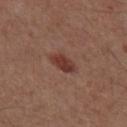Imaged during a routine full-body skin examination; the lesion was not biopsied and no histopathology is available.
The lesion is located on the front of the torso.
A male patient, aged 53 to 57.
The lesion's longest dimension is about 3.5 mm.
The tile uses white-light illumination.
A lesion tile, about 15 mm wide, cut from a 3D total-body photograph.
The total-body-photography lesion software estimated border irregularity of about 2 on a 0–10 scale, a within-lesion color-variation index near 2/10, and peripheral color asymmetry of about 0.5. The software also gave a nevus-likeness score of about 95/100 and a lesion-detection confidence of about 100/100.